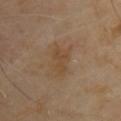Impression: No biopsy was performed on this lesion — it was imaged during a full skin examination and was not determined to be concerning. Clinical summary: Cropped from a total-body skin-imaging series; the visible field is about 15 mm. Automated tile analysis of the lesion measured a lesion area of about 5.5 mm² and two-axis asymmetry of about 0.45. And it measured an average lesion color of about L≈45 a*≈15 b*≈31 (CIELAB), about 6 CIELAB-L* units darker than the surrounding skin, and a lesion-to-skin contrast of about 5.5 (normalized; higher = more distinct). It also reported a border-irregularity index near 5/10, a within-lesion color-variation index near 1.5/10, and a peripheral color-asymmetry measure near 0.5. Longest diameter approximately 3.5 mm. Imaged with cross-polarized lighting. From the right upper arm. A male subject, in their mid- to late 60s.